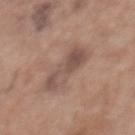notes: imaged on a skin check; not biopsied
illumination: white-light illumination
imaging modality: 15 mm crop, total-body photography
body site: the upper back
diameter: about 5.5 mm
patient: female, aged approximately 60